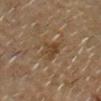The lesion was tiled from a total-body skin photograph and was not biopsied. About 3.5 mm across. A male patient, aged 68 to 72. Located on the head or neck. An algorithmic analysis of the crop reported an eccentricity of roughly 0.8 and two-axis asymmetry of about 0.35. The software also gave a lesion color around L≈39 a*≈15 b*≈30 in CIELAB, about 7 CIELAB-L* units darker than the surrounding skin, and a lesion-to-skin contrast of about 6.5 (normalized; higher = more distinct). It also reported a border-irregularity index near 4/10, a color-variation rating of about 2.5/10, and a peripheral color-asymmetry measure near 0.5. The tile uses cross-polarized illumination. Cropped from a whole-body photographic skin survey; the tile spans about 15 mm.